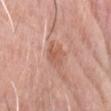The lesion was photographed on a routine skin check and not biopsied; there is no pathology result.
From the head or neck.
A male patient approximately 80 years of age.
The recorded lesion diameter is about 3 mm.
The lesion-visualizer software estimated a footprint of about 5.5 mm², an outline eccentricity of about 0.65 (0 = round, 1 = elongated), and two-axis asymmetry of about 0.25. The analysis additionally found border irregularity of about 3 on a 0–10 scale and peripheral color asymmetry of about 1. The analysis additionally found a classifier nevus-likeness of about 0/100 and a detector confidence of about 100 out of 100 that the crop contains a lesion.
A close-up tile cropped from a whole-body skin photograph, about 15 mm across.
Captured under white-light illumination.